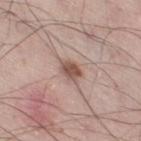Case summary:
– follow-up — imaged on a skin check; not biopsied
– lighting — white-light illumination
– image source — 15 mm crop, total-body photography
– diameter — about 3 mm
– location — the right thigh
– subject — male, in their mid- to late 50s